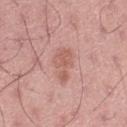Assessment: Imaged during a routine full-body skin examination; the lesion was not biopsied and no histopathology is available. Clinical summary: The recorded lesion diameter is about 3.5 mm. The lesion-visualizer software estimated a footprint of about 6 mm², an eccentricity of roughly 0.8, and a symmetry-axis asymmetry near 0.5. The software also gave an average lesion color of about L≈58 a*≈24 b*≈27 (CIELAB) and roughly 8 lightness units darker than nearby skin. The tile uses white-light illumination. A male subject, aged around 45. Located on the right thigh. A 15 mm close-up tile from a total-body photography series done for melanoma screening.